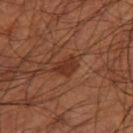Part of a total-body skin-imaging series; this lesion was reviewed on a skin check and was not flagged for biopsy. The lesion is located on the right thigh. An algorithmic analysis of the crop reported a lesion area of about 4.5 mm² and a symmetry-axis asymmetry near 0.3. And it measured about 8 CIELAB-L* units darker than the surrounding skin and a normalized lesion–skin contrast near 7.5. And it measured border irregularity of about 3 on a 0–10 scale, a color-variation rating of about 1.5/10, and a peripheral color-asymmetry measure near 0.5. And it measured an automated nevus-likeness rating near 45 out of 100 and a lesion-detection confidence of about 100/100. Imaged with cross-polarized lighting. The subject is a male aged approximately 60. Approximately 3 mm at its widest. Cropped from a total-body skin-imaging series; the visible field is about 15 mm.